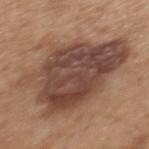Impression: The lesion was photographed on a routine skin check and not biopsied; there is no pathology result.